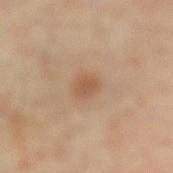The lesion was tiled from a total-body skin photograph and was not biopsied. This is a cross-polarized tile. This image is a 15 mm lesion crop taken from a total-body photograph. A male subject, aged around 65. Longest diameter approximately 2.5 mm. On the lower back.